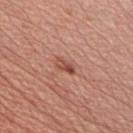This lesion was catalogued during total-body skin photography and was not selected for biopsy. The total-body-photography lesion software estimated a lesion area of about 2.5 mm², a shape eccentricity near 0.9, and a symmetry-axis asymmetry near 0.35. The software also gave border irregularity of about 4.5 on a 0–10 scale, internal color variation of about 1 on a 0–10 scale, and peripheral color asymmetry of about 0. A 15 mm crop from a total-body photograph taken for skin-cancer surveillance. This is a white-light tile. The lesion is on the chest. The subject is a male aged approximately 65.